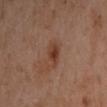workup: catalogued during a skin exam; not biopsied | site: the left forearm | lesion size: about 3 mm | image-analysis metrics: a lesion area of about 4.5 mm², an eccentricity of roughly 0.75, and a shape-asymmetry score of about 0.25 (0 = symmetric); a mean CIELAB color near L≈40 a*≈22 b*≈29, about 9 CIELAB-L* units darker than the surrounding skin, and a normalized lesion–skin contrast near 8; a border-irregularity index near 2.5/10, internal color variation of about 4 on a 0–10 scale, and peripheral color asymmetry of about 1; a nevus-likeness score of about 80/100 and lesion-presence confidence of about 100/100 | image source: ~15 mm tile from a whole-body skin photo | subject: female, aged 38–42.